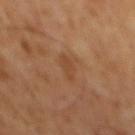Case summary:
– workup: no biopsy performed (imaged during a skin exam)
– image source: 15 mm crop, total-body photography
– subject: male, approximately 65 years of age
– lighting: cross-polarized illumination